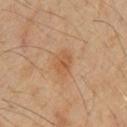Image and clinical context: Cropped from a whole-body photographic skin survey; the tile spans about 15 mm. A male patient, roughly 60 years of age. Longest diameter approximately 3 mm. Located on the chest.